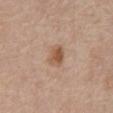No biopsy was performed on this lesion — it was imaged during a full skin examination and was not determined to be concerning. The total-body-photography lesion software estimated an area of roughly 5 mm². It also reported internal color variation of about 4 on a 0–10 scale and radial color variation of about 1.5. The software also gave a nevus-likeness score of about 85/100 and a lesion-detection confidence of about 100/100. Captured under white-light illumination. The lesion's longest dimension is about 2.5 mm. A region of skin cropped from a whole-body photographic capture, roughly 15 mm wide. A male subject in their mid- to late 60s. The lesion is located on the abdomen.